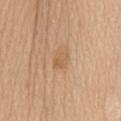A female patient roughly 70 years of age. A lesion tile, about 15 mm wide, cut from a 3D total-body photograph. Longest diameter approximately 2.5 mm. On the mid back. This is a white-light tile. The lesion-visualizer software estimated a lesion area of about 4 mm², an eccentricity of roughly 0.7, and two-axis asymmetry of about 0.2.